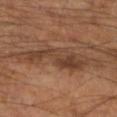Acquisition and patient details:
A 15 mm crop from a total-body photograph taken for skin-cancer surveillance. The total-body-photography lesion software estimated a lesion-detection confidence of about 100/100. On the left forearm. A male patient aged around 65. The recorded lesion diameter is about 7 mm. The tile uses cross-polarized illumination.